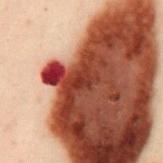Captured during whole-body skin photography for melanoma surveillance; the lesion was not biopsied.
A 15 mm close-up tile from a total-body photography series done for melanoma screening.
The patient is a male aged approximately 50.
The lesion is on the back.
An algorithmic analysis of the crop reported a lesion area of about 2.5 mm² and a shape eccentricity near 0.7. The analysis additionally found an average lesion color of about L≈29 a*≈27 b*≈27 (CIELAB), a lesion–skin lightness drop of about 8, and a lesion-to-skin contrast of about 8 (normalized; higher = more distinct).
Captured under cross-polarized illumination.